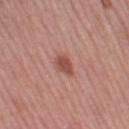Impression:
No biopsy was performed on this lesion — it was imaged during a full skin examination and was not determined to be concerning.
Clinical summary:
A female patient, aged approximately 45. Automated tile analysis of the lesion measured an area of roughly 4.5 mm², an eccentricity of roughly 0.65, and two-axis asymmetry of about 0.25. It also reported border irregularity of about 2 on a 0–10 scale, internal color variation of about 2 on a 0–10 scale, and peripheral color asymmetry of about 0.5. And it measured an automated nevus-likeness rating near 90 out of 100 and a lesion-detection confidence of about 100/100. A 15 mm close-up extracted from a 3D total-body photography capture. On the left thigh.